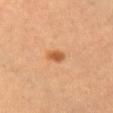This lesion was catalogued during total-body skin photography and was not selected for biopsy.
The lesion is on the left thigh.
A female subject aged 58 to 62.
Captured under cross-polarized illumination.
A roughly 15 mm field-of-view crop from a total-body skin photograph.